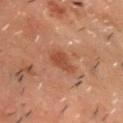Imaged during a routine full-body skin examination; the lesion was not biopsied and no histopathology is available. A 15 mm close-up extracted from a 3D total-body photography capture. The tile uses cross-polarized illumination. The subject is a male approximately 50 years of age. Located on the head or neck.